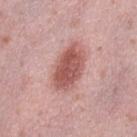Assessment:
This lesion was catalogued during total-body skin photography and was not selected for biopsy.
Acquisition and patient details:
A female patient, aged 38 to 42. Automated tile analysis of the lesion measured a lesion color around L≈55 a*≈26 b*≈24 in CIELAB. The analysis additionally found a border-irregularity index near 2/10, internal color variation of about 4 on a 0–10 scale, and a peripheral color-asymmetry measure near 1.5. It also reported a classifier nevus-likeness of about 90/100 and a lesion-detection confidence of about 100/100. A lesion tile, about 15 mm wide, cut from a 3D total-body photograph. The tile uses white-light illumination. From the left lower leg.